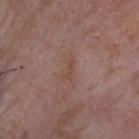| feature | finding |
|---|---|
| workup | total-body-photography surveillance lesion; no biopsy |
| TBP lesion metrics | a nevus-likeness score of about 0/100 and a lesion-detection confidence of about 100/100 |
| subject | female, aged 83 or older |
| tile lighting | white-light illumination |
| image | 15 mm crop, total-body photography |
| location | the head or neck |